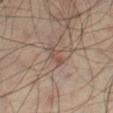This lesion was catalogued during total-body skin photography and was not selected for biopsy.
The tile uses cross-polarized illumination.
A region of skin cropped from a whole-body photographic capture, roughly 15 mm wide.
Approximately 3 mm at its widest.
The lesion is on the right thigh.
The lesion-visualizer software estimated a mean CIELAB color near L≈47 a*≈17 b*≈23 and a normalized lesion–skin contrast near 5.5. The software also gave a classifier nevus-likeness of about 0/100 and lesion-presence confidence of about 95/100.
A male subject, about 60 years old.